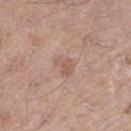Clinical impression: Part of a total-body skin-imaging series; this lesion was reviewed on a skin check and was not flagged for biopsy. Background: The patient is a male aged approximately 60. The total-body-photography lesion software estimated roughly 7 lightness units darker than nearby skin. About 2.5 mm across. From the left thigh. A 15 mm close-up extracted from a 3D total-body photography capture.